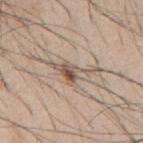Assessment:
No biopsy was performed on this lesion — it was imaged during a full skin examination and was not determined to be concerning.